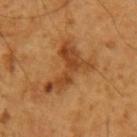biopsy status = catalogued during a skin exam; not biopsied
acquisition = ~15 mm crop, total-body skin-cancer survey
location = the right upper arm
size = ≈6 mm
image-analysis metrics = a border-irregularity rating of about 9/10, internal color variation of about 4.5 on a 0–10 scale, and peripheral color asymmetry of about 1.5; a nevus-likeness score of about 5/100 and a lesion-detection confidence of about 100/100
subject = male, roughly 60 years of age
tile lighting = cross-polarized illumination